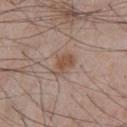workup=total-body-photography surveillance lesion; no biopsy | patient=male, in their mid-60s | body site=the chest | image=~15 mm tile from a whole-body skin photo.